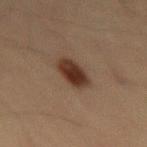Q: Was a biopsy performed?
A: imaged on a skin check; not biopsied
Q: Lesion location?
A: the right thigh
Q: Patient demographics?
A: male, aged 68 to 72
Q: Illumination type?
A: cross-polarized
Q: Automated lesion metrics?
A: a color-variation rating of about 3.5/10 and peripheral color asymmetry of about 1
Q: What is the imaging modality?
A: total-body-photography crop, ~15 mm field of view